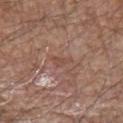Clinical impression:
This lesion was catalogued during total-body skin photography and was not selected for biopsy.
Acquisition and patient details:
Located on the left upper arm. A male subject aged 78–82. Cropped from a total-body skin-imaging series; the visible field is about 15 mm.